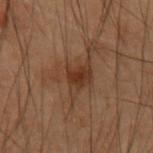{"biopsy_status": "not biopsied; imaged during a skin examination", "patient": {"sex": "male", "age_approx": 55}, "lighting": "cross-polarized", "image": {"source": "total-body photography crop", "field_of_view_mm": 15}, "site": "right forearm"}